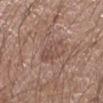Assessment:
Imaged during a routine full-body skin examination; the lesion was not biopsied and no histopathology is available.
Context:
The patient is a male about 65 years old. Imaged with white-light lighting. Located on the right upper arm. Measured at roughly 3.5 mm in maximum diameter. A roughly 15 mm field-of-view crop from a total-body skin photograph. The lesion-visualizer software estimated an average lesion color of about L≈49 a*≈18 b*≈24 (CIELAB), about 7 CIELAB-L* units darker than the surrounding skin, and a normalized lesion–skin contrast near 5. It also reported a border-irregularity index near 4/10, a within-lesion color-variation index near 3/10, and a peripheral color-asymmetry measure near 1. The software also gave a classifier nevus-likeness of about 10/100 and lesion-presence confidence of about 100/100.